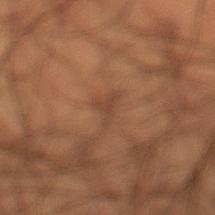workup: imaged on a skin check; not biopsied
acquisition: total-body-photography crop, ~15 mm field of view
subject: male, aged 48–52
site: the left lower leg